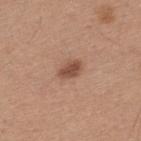Impression:
Recorded during total-body skin imaging; not selected for excision or biopsy.
Image and clinical context:
The subject is a male aged 28–32. Automated image analysis of the tile measured an eccentricity of roughly 0.75 and a shape-asymmetry score of about 0.25 (0 = symmetric). The software also gave a border-irregularity index near 2/10, internal color variation of about 2 on a 0–10 scale, and peripheral color asymmetry of about 0.5. Cropped from a total-body skin-imaging series; the visible field is about 15 mm. The lesion is on the upper back.